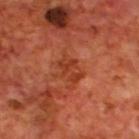biopsy status = imaged on a skin check; not biopsied
imaging modality = 15 mm crop, total-body photography
subject = male, aged 68–72
body site = the upper back
size = about 3.5 mm